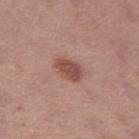workup = no biopsy performed (imaged during a skin exam) | patient = female, aged 38–42 | location = the leg | acquisition = total-body-photography crop, ~15 mm field of view | size = about 3.5 mm | TBP lesion metrics = a border-irregularity index near 2/10 and radial color variation of about 0.5; an automated nevus-likeness rating near 90 out of 100 and lesion-presence confidence of about 100/100 | tile lighting = white-light.